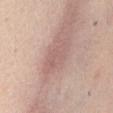Captured during whole-body skin photography for melanoma surveillance; the lesion was not biopsied.
Located on the abdomen.
Approximately 4.5 mm at its widest.
The patient is a female aged 43 to 47.
This image is a 15 mm lesion crop taken from a total-body photograph.
Imaged with white-light lighting.